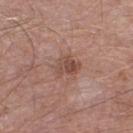  biopsy_status: not biopsied; imaged during a skin examination
  image:
    source: total-body photography crop
    field_of_view_mm: 15
  patient:
    sex: male
    age_approx: 55
  automated_metrics:
    nevus_likeness_0_100: 10
  site: left lower leg
  lesion_size:
    long_diameter_mm_approx: 3.0
  lighting: white-light